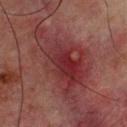follow-up: total-body-photography surveillance lesion; no biopsy | patient: male, approximately 65 years of age | image source: ~15 mm crop, total-body skin-cancer survey | anatomic site: the back.